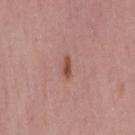- biopsy status — catalogued during a skin exam; not biopsied
- subject — female, roughly 50 years of age
- tile lighting — white-light illumination
- size — ~2.5 mm (longest diameter)
- imaging modality — ~15 mm tile from a whole-body skin photo
- body site — the leg